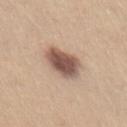No biopsy was performed on this lesion — it was imaged during a full skin examination and was not determined to be concerning.
Located on the abdomen.
A female subject, aged 23–27.
A region of skin cropped from a whole-body photographic capture, roughly 15 mm wide.
The lesion-visualizer software estimated a footprint of about 11 mm², a shape eccentricity near 0.7, and a symmetry-axis asymmetry near 0.2. And it measured a mean CIELAB color near L≈53 a*≈18 b*≈26, a lesion–skin lightness drop of about 17, and a normalized border contrast of about 11. It also reported border irregularity of about 2 on a 0–10 scale, a within-lesion color-variation index near 4.5/10, and radial color variation of about 1.5.
Measured at roughly 4.5 mm in maximum diameter.
The tile uses white-light illumination.